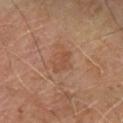Assessment:
The lesion was tiled from a total-body skin photograph and was not biopsied.
Clinical summary:
This is a cross-polarized tile. Cropped from a total-body skin-imaging series; the visible field is about 15 mm. From the right leg. A male patient, in their 60s. The lesion's longest dimension is about 3 mm.